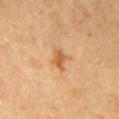Clinical summary:
A 15 mm close-up extracted from a 3D total-body photography capture. A female patient, approximately 50 years of age. From the mid back. Imaged with cross-polarized lighting. The lesion's longest dimension is about 2.5 mm. The lesion-visualizer software estimated an eccentricity of roughly 0.85 and a shape-asymmetry score of about 0.35 (0 = symmetric).